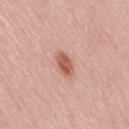No biopsy was performed on this lesion — it was imaged during a full skin examination and was not determined to be concerning.
A region of skin cropped from a whole-body photographic capture, roughly 15 mm wide.
The subject is a female about 70 years old.
Measured at roughly 3.5 mm in maximum diameter.
Located on the lower back.